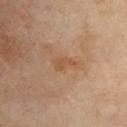Findings:
* biopsy status · no biopsy performed (imaged during a skin exam)
* acquisition · ~15 mm tile from a whole-body skin photo
* subject · female, aged around 50
* location · the chest
* lesion diameter · ~2.5 mm (longest diameter)
* TBP lesion metrics · a footprint of about 3 mm², an outline eccentricity of about 0.85 (0 = round, 1 = elongated), and a symmetry-axis asymmetry near 0.45; an average lesion color of about L≈50 a*≈20 b*≈34 (CIELAB), about 6 CIELAB-L* units darker than the surrounding skin, and a lesion-to-skin contrast of about 5.5 (normalized; higher = more distinct); a nevus-likeness score of about 0/100 and lesion-presence confidence of about 100/100